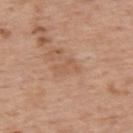biopsy status — total-body-photography surveillance lesion; no biopsy
imaging modality — 15 mm crop, total-body photography
lesion size — ≈3 mm
tile lighting — white-light illumination
automated metrics — a nevus-likeness score of about 0/100 and lesion-presence confidence of about 100/100
subject — male, roughly 75 years of age
site — the upper back